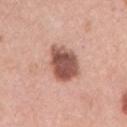follow-up=imaged on a skin check; not biopsied | anatomic site=the right upper arm | image source=total-body-photography crop, ~15 mm field of view | lighting=white-light | lesion size=~4.5 mm (longest diameter) | subject=female, approximately 60 years of age.